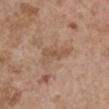biopsy status — total-body-photography surveillance lesion; no biopsy | subject — female, aged 63–67 | acquisition — 15 mm crop, total-body photography | lighting — white-light illumination | size — ~4.5 mm (longest diameter) | automated metrics — a shape eccentricity near 0.9 and a shape-asymmetry score of about 0.5 (0 = symmetric); a lesion color around L≈53 a*≈18 b*≈30 in CIELAB, a lesion–skin lightness drop of about 7, and a lesion-to-skin contrast of about 5.5 (normalized; higher = more distinct); a border-irregularity rating of about 6/10, internal color variation of about 2 on a 0–10 scale, and radial color variation of about 0.5; a nevus-likeness score of about 0/100 and a detector confidence of about 100 out of 100 that the crop contains a lesion | location — the right upper arm.